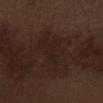The lesion was photographed on a routine skin check and not biopsied; there is no pathology result. Automated image analysis of the tile measured a footprint of about 17 mm² and a shape-asymmetry score of about 0.25 (0 = symmetric). It also reported a border-irregularity rating of about 4/10, a color-variation rating of about 2/10, and peripheral color asymmetry of about 1. Cropped from a total-body skin-imaging series; the visible field is about 15 mm. This is a white-light tile. The lesion is located on the abdomen. A male subject about 70 years old.